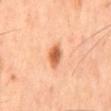Cropped from a total-body skin-imaging series; the visible field is about 15 mm. Measured at roughly 3.5 mm in maximum diameter. Imaged with cross-polarized lighting. The patient is a male in their mid-40s. From the mid back.